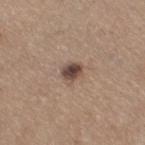Imaged during a routine full-body skin examination; the lesion was not biopsied and no histopathology is available. A male patient, aged 63–67. The lesion is located on the right thigh. Longest diameter approximately 3 mm. A 15 mm close-up tile from a total-body photography series done for melanoma screening. The tile uses white-light illumination.